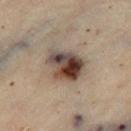notes: total-body-photography surveillance lesion; no biopsy
TBP lesion metrics: an outline eccentricity of about 0.65 (0 = round, 1 = elongated); an automated nevus-likeness rating near 95 out of 100 and a detector confidence of about 100 out of 100 that the crop contains a lesion
location: the leg
subject: female, about 60 years old
lesion diameter: about 4.5 mm
lighting: cross-polarized
image: ~15 mm tile from a whole-body skin photo